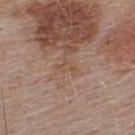tile lighting=white-light illumination | body site=the back | subject=male, aged 53–57 | acquisition=15 mm crop, total-body photography.